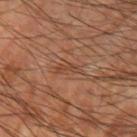| key | value |
|---|---|
| workup | no biopsy performed (imaged during a skin exam) |
| image | total-body-photography crop, ~15 mm field of view |
| lesion diameter | about 2.5 mm |
| patient | male, roughly 60 years of age |
| body site | the left upper arm |
| lighting | cross-polarized |
| image-analysis metrics | a lesion color around L≈43 a*≈21 b*≈30 in CIELAB; a classifier nevus-likeness of about 0/100 and a lesion-detection confidence of about 85/100 |